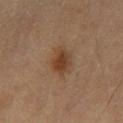Clinical impression: Imaged during a routine full-body skin examination; the lesion was not biopsied and no histopathology is available.